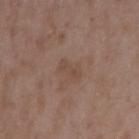<case>
<image>
  <source>total-body photography crop</source>
  <field_of_view_mm>15</field_of_view_mm>
</image>
<site>arm</site>
<patient>
  <sex>male</sex>
  <age_approx>50</age_approx>
</patient>
</case>